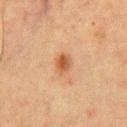Q: Is there a histopathology result?
A: catalogued during a skin exam; not biopsied
Q: Lesion size?
A: about 3 mm
Q: What lighting was used for the tile?
A: cross-polarized illumination
Q: Where on the body is the lesion?
A: the chest
Q: Patient demographics?
A: male, approximately 50 years of age
Q: What kind of image is this?
A: ~15 mm crop, total-body skin-cancer survey